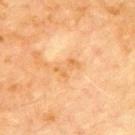workup=total-body-photography surveillance lesion; no biopsy | patient=male, aged 68–72 | lesion diameter=~3 mm (longest diameter) | site=the front of the torso | image=~15 mm crop, total-body skin-cancer survey.